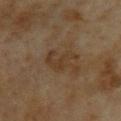biopsy status — total-body-photography surveillance lesion; no biopsy | body site — the upper back | image source — ~15 mm crop, total-body skin-cancer survey | patient — female, in their 60s | automated metrics — a lesion area of about 7 mm², a shape eccentricity near 0.75, and a symmetry-axis asymmetry near 0.5; a classifier nevus-likeness of about 0/100 and lesion-presence confidence of about 100/100 | illumination — cross-polarized illumination.